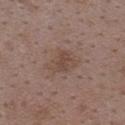acquisition — 15 mm crop, total-body photography; subject — female, approximately 35 years of age; site — the upper back.